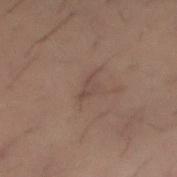Background: On the right forearm. The lesion-visualizer software estimated a footprint of about 2.5 mm², an eccentricity of roughly 0.85, and a shape-asymmetry score of about 0.5 (0 = symmetric). It also reported border irregularity of about 5.5 on a 0–10 scale and radial color variation of about 0. The software also gave lesion-presence confidence of about 70/100. Approximately 3 mm at its widest. A 15 mm close-up extracted from a 3D total-body photography capture. Captured under cross-polarized illumination. A male subject approximately 30 years of age.